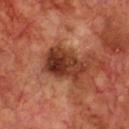This lesion was catalogued during total-body skin photography and was not selected for biopsy.
A male patient aged 68–72.
A 15 mm crop from a total-body photograph taken for skin-cancer surveillance.
Measured at roughly 5.5 mm in maximum diameter.
The lesion is on the front of the torso.
The total-body-photography lesion software estimated a footprint of about 15 mm², a shape eccentricity near 0.8, and a symmetry-axis asymmetry near 0.25. And it measured a mean CIELAB color near L≈35 a*≈26 b*≈30 and a lesion–skin lightness drop of about 14. And it measured a border-irregularity index near 3/10, internal color variation of about 8.5 on a 0–10 scale, and radial color variation of about 3.